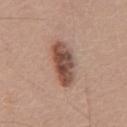Q: Is there a histopathology result?
A: catalogued during a skin exam; not biopsied
Q: Lesion location?
A: the front of the torso
Q: What lighting was used for the tile?
A: white-light illumination
Q: Who is the patient?
A: male, aged approximately 60
Q: What is the lesion's diameter?
A: ~5.5 mm (longest diameter)
Q: How was this image acquired?
A: 15 mm crop, total-body photography